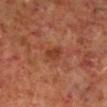Q: Was this lesion biopsied?
A: catalogued during a skin exam; not biopsied
Q: Who is the patient?
A: male, aged around 60
Q: What is the imaging modality?
A: 15 mm crop, total-body photography
Q: Where on the body is the lesion?
A: the right lower leg